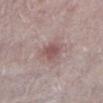Assessment: No biopsy was performed on this lesion — it was imaged during a full skin examination and was not determined to be concerning. Image and clinical context: A close-up tile cropped from a whole-body skin photograph, about 15 mm across. Automated tile analysis of the lesion measured a footprint of about 5.5 mm², a shape eccentricity near 0.45, and a shape-asymmetry score of about 0.25 (0 = symmetric). And it measured roughly 10 lightness units darker than nearby skin. The patient is a female roughly 60 years of age. About 2.5 mm across. This is a white-light tile. From the left lower leg.